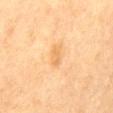Assessment: This lesion was catalogued during total-body skin photography and was not selected for biopsy. Context: Automated tile analysis of the lesion measured a lesion area of about 3 mm². The software also gave a border-irregularity index near 2.5/10, internal color variation of about 0.5 on a 0–10 scale, and peripheral color asymmetry of about 0. And it measured a classifier nevus-likeness of about 5/100 and a detector confidence of about 100 out of 100 that the crop contains a lesion. From the mid back. A 15 mm crop from a total-body photograph taken for skin-cancer surveillance. Approximately 2.5 mm at its widest. Captured under cross-polarized illumination. A female patient aged 53 to 57.